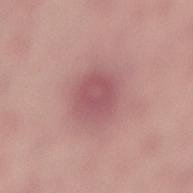<lesion>
  <biopsy_status>not biopsied; imaged during a skin examination</biopsy_status>
</lesion>